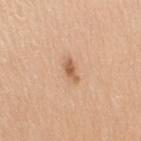notes: total-body-photography surveillance lesion; no biopsy | lighting: white-light illumination | location: the right upper arm | image source: 15 mm crop, total-body photography | patient: male, aged 38–42 | diameter: ≈3 mm | automated lesion analysis: a footprint of about 3 mm² and a shape-asymmetry score of about 0.35 (0 = symmetric).